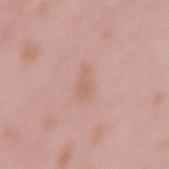- follow-up: no biopsy performed (imaged during a skin exam)
- patient: female, aged approximately 15
- site: the arm
- lighting: white-light illumination
- acquisition: ~15 mm crop, total-body skin-cancer survey